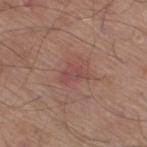- follow-up — catalogued during a skin exam; not biopsied
- anatomic site — the leg
- image — ~15 mm tile from a whole-body skin photo
- lesion diameter — about 3 mm
- image-analysis metrics — an eccentricity of roughly 0.8 and a shape-asymmetry score of about 0.35 (0 = symmetric); a mean CIELAB color near L≈47 a*≈24 b*≈22, about 6 CIELAB-L* units darker than the surrounding skin, and a normalized border contrast of about 5; a border-irregularity index near 3.5/10, a within-lesion color-variation index near 2.5/10, and a peripheral color-asymmetry measure near 1
- patient — male, in their 80s